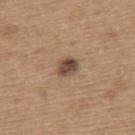Clinical impression: This lesion was catalogued during total-body skin photography and was not selected for biopsy. Context: The lesion is on the upper back. The patient is a male roughly 65 years of age. Approximately 2.5 mm at its widest. A roughly 15 mm field-of-view crop from a total-body skin photograph.